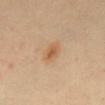follow-up: imaged on a skin check; not biopsied | subject: female, about 35 years old | lighting: cross-polarized | location: the abdomen | diameter: ≈3 mm | imaging modality: ~15 mm crop, total-body skin-cancer survey.